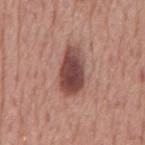The lesion was tiled from a total-body skin photograph and was not biopsied. A male patient, aged approximately 75. About 5.5 mm across. The lesion is located on the mid back. A 15 mm close-up extracted from a 3D total-body photography capture. Automated image analysis of the tile measured internal color variation of about 5 on a 0–10 scale and peripheral color asymmetry of about 1.5. And it measured a nevus-likeness score of about 95/100 and a lesion-detection confidence of about 100/100.